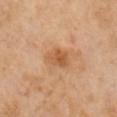workup: imaged on a skin check; not biopsied
tile lighting: cross-polarized
automated metrics: a within-lesion color-variation index near 3/10 and a peripheral color-asymmetry measure near 1; a lesion-detection confidence of about 100/100
patient: male, in their mid-60s
acquisition: ~15 mm tile from a whole-body skin photo
lesion size: ≈3 mm